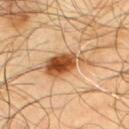This lesion was catalogued during total-body skin photography and was not selected for biopsy.
From the upper back.
This image is a 15 mm lesion crop taken from a total-body photograph.
The subject is a male aged 63–67.